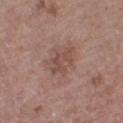Imaged with white-light lighting.
Cropped from a whole-body photographic skin survey; the tile spans about 15 mm.
A female subject, aged 38–42.
On the right thigh.
About 3.5 mm across.